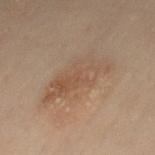Context:
A male subject aged approximately 50. Approximately 7 mm at its widest. The tile uses cross-polarized illumination. Cropped from a total-body skin-imaging series; the visible field is about 15 mm. The lesion is on the mid back.